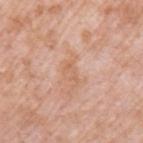This lesion was catalogued during total-body skin photography and was not selected for biopsy.
The subject is a male aged 48–52.
The lesion is located on the left upper arm.
About 2.5 mm across.
Cropped from a whole-body photographic skin survey; the tile spans about 15 mm.